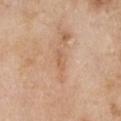| field | value |
|---|---|
| workup | catalogued during a skin exam; not biopsied |
| size | ≈2.5 mm |
| illumination | white-light illumination |
| acquisition | ~15 mm tile from a whole-body skin photo |
| patient | female, aged around 65 |
| anatomic site | the chest |